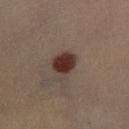Clinical impression:
This lesion was catalogued during total-body skin photography and was not selected for biopsy.
Acquisition and patient details:
A male patient, aged 38 to 42. On the right lower leg. This is a cross-polarized tile. An algorithmic analysis of the crop reported a lesion area of about 7.5 mm², an eccentricity of roughly 0.65, and two-axis asymmetry of about 0.25. And it measured border irregularity of about 2 on a 0–10 scale, internal color variation of about 3 on a 0–10 scale, and peripheral color asymmetry of about 1. This image is a 15 mm lesion crop taken from a total-body photograph.